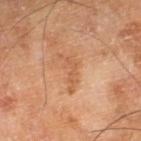Clinical impression:
This lesion was catalogued during total-body skin photography and was not selected for biopsy.
Acquisition and patient details:
On the right lower leg. Imaged with cross-polarized lighting. The lesion-visualizer software estimated an area of roughly 4.5 mm². It also reported an average lesion color of about L≈59 a*≈24 b*≈38 (CIELAB), about 8 CIELAB-L* units darker than the surrounding skin, and a lesion-to-skin contrast of about 5.5 (normalized; higher = more distinct). And it measured a border-irregularity index near 9.5/10, a within-lesion color-variation index near 0.5/10, and radial color variation of about 0. A 15 mm close-up tile from a total-body photography series done for melanoma screening. A male patient, roughly 65 years of age.